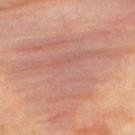Impression:
Imaged during a routine full-body skin examination; the lesion was not biopsied and no histopathology is available.
Acquisition and patient details:
On the upper back. A male patient, about 35 years old. Imaged with cross-polarized lighting. A region of skin cropped from a whole-body photographic capture, roughly 15 mm wide. About 13 mm across.